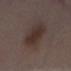A region of skin cropped from a whole-body photographic capture, roughly 15 mm wide. The lesion is located on the left lower leg. A male subject aged 68–72.